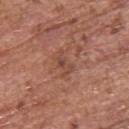Q: Was this lesion biopsied?
A: no biopsy performed (imaged during a skin exam)
Q: What is the imaging modality?
A: ~15 mm crop, total-body skin-cancer survey
Q: How was the tile lit?
A: white-light illumination
Q: Patient demographics?
A: female, aged 63–67
Q: Where on the body is the lesion?
A: the upper back